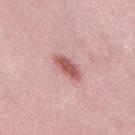biopsy status — catalogued during a skin exam; not biopsied
image — total-body-photography crop, ~15 mm field of view
lesion size — ~4 mm (longest diameter)
site — the right thigh
subject — female, aged approximately 40
lighting — white-light illumination
TBP lesion metrics — a footprint of about 5.5 mm², a shape eccentricity near 0.9, and a shape-asymmetry score of about 0.25 (0 = symmetric); a lesion color around L≈57 a*≈26 b*≈25 in CIELAB, roughly 13 lightness units darker than nearby skin, and a normalized border contrast of about 9; border irregularity of about 3 on a 0–10 scale and a color-variation rating of about 2.5/10; a classifier nevus-likeness of about 95/100